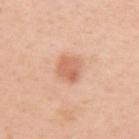Q: Is there a histopathology result?
A: imaged on a skin check; not biopsied
Q: Where on the body is the lesion?
A: the right upper arm
Q: Who is the patient?
A: female, in their mid- to late 40s
Q: How was the tile lit?
A: white-light
Q: Lesion size?
A: about 3 mm
Q: How was this image acquired?
A: total-body-photography crop, ~15 mm field of view